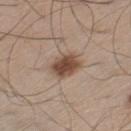Q: Is there a histopathology result?
A: no biopsy performed (imaged during a skin exam)
Q: What is the lesion's diameter?
A: ≈3.5 mm
Q: Where on the body is the lesion?
A: the left thigh
Q: Illumination type?
A: white-light
Q: Automated lesion metrics?
A: a peripheral color-asymmetry measure near 1
Q: What is the imaging modality?
A: ~15 mm crop, total-body skin-cancer survey
Q: What are the patient's age and sex?
A: male, aged 58 to 62